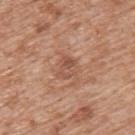Q: Is there a histopathology result?
A: no biopsy performed (imaged during a skin exam)
Q: What lighting was used for the tile?
A: white-light illumination
Q: What is the anatomic site?
A: the upper back
Q: Patient demographics?
A: male, in their 60s
Q: How large is the lesion?
A: ~2.5 mm (longest diameter)
Q: How was this image acquired?
A: ~15 mm tile from a whole-body skin photo